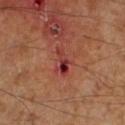Imaged during a routine full-body skin examination; the lesion was not biopsied and no histopathology is available.
The tile uses cross-polarized illumination.
A male subject aged 58–62.
From the left lower leg.
Longest diameter approximately 3 mm.
A 15 mm close-up extracted from a 3D total-body photography capture.